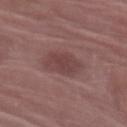| feature | finding |
|---|---|
| follow-up | no biopsy performed (imaged during a skin exam) |
| image source | ~15 mm crop, total-body skin-cancer survey |
| subject | female, approximately 70 years of age |
| site | the left forearm |
| automated lesion analysis | a lesion area of about 9.5 mm² and a symmetry-axis asymmetry near 0.15; an average lesion color of about L≈42 a*≈21 b*≈19 (CIELAB) and roughly 8 lightness units darker than nearby skin; border irregularity of about 2 on a 0–10 scale, a within-lesion color-variation index near 2/10, and radial color variation of about 0.5; a classifier nevus-likeness of about 10/100 and a lesion-detection confidence of about 100/100 |
| size | ≈4 mm |
| tile lighting | white-light |